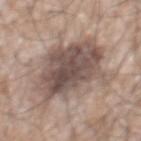biopsy status: no biopsy performed (imaged during a skin exam)
size: ≈8 mm
site: the mid back
subject: male, aged approximately 60
acquisition: ~15 mm tile from a whole-body skin photo
automated lesion analysis: an average lesion color of about L≈51 a*≈14 b*≈21 (CIELAB), about 13 CIELAB-L* units darker than the surrounding skin, and a lesion-to-skin contrast of about 9.5 (normalized; higher = more distinct); a border-irregularity rating of about 5/10, internal color variation of about 6 on a 0–10 scale, and radial color variation of about 2